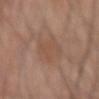{"biopsy_status": "not biopsied; imaged during a skin examination", "patient": {"sex": "male", "age_approx": 55}, "image": {"source": "total-body photography crop", "field_of_view_mm": 15}, "site": "right forearm"}